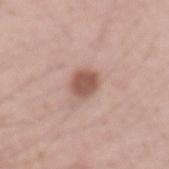No biopsy was performed on this lesion — it was imaged during a full skin examination and was not determined to be concerning. Cropped from a total-body skin-imaging series; the visible field is about 15 mm. A male patient, aged approximately 55. Measured at roughly 3 mm in maximum diameter. This is a white-light tile. The lesion is located on the left forearm.